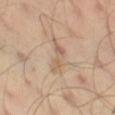Clinical impression:
The lesion was photographed on a routine skin check and not biopsied; there is no pathology result.
Acquisition and patient details:
A 15 mm close-up tile from a total-body photography series done for melanoma screening. From the left thigh. A male subject, about 45 years old. The recorded lesion diameter is about 4.5 mm. The tile uses cross-polarized illumination. An algorithmic analysis of the crop reported internal color variation of about 5 on a 0–10 scale and radial color variation of about 1.5.